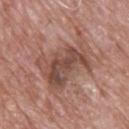follow-up — catalogued during a skin exam; not biopsied
lighting — white-light
image — total-body-photography crop, ~15 mm field of view
anatomic site — the upper back
automated lesion analysis — an area of roughly 25 mm²; a lesion color around L≈49 a*≈21 b*≈25 in CIELAB and a lesion-to-skin contrast of about 7.5 (normalized; higher = more distinct); a nevus-likeness score of about 0/100
diameter — ~8 mm (longest diameter)
patient — male, aged 68 to 72